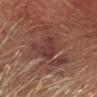biopsy status — catalogued during a skin exam; not biopsied
patient — male, approximately 75 years of age
lesion size — ≈4 mm
lighting — cross-polarized illumination
automated metrics — a shape eccentricity near 0.85; a classifier nevus-likeness of about 0/100 and lesion-presence confidence of about 80/100
anatomic site — the head or neck
image — ~15 mm tile from a whole-body skin photo